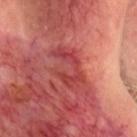follow-up: catalogued during a skin exam; not biopsied
image: total-body-photography crop, ~15 mm field of view
automated lesion analysis: a symmetry-axis asymmetry near 0.5; about 8 CIELAB-L* units darker than the surrounding skin; an automated nevus-likeness rating near 0 out of 100
illumination: cross-polarized illumination
anatomic site: the head or neck
subject: male, aged 58 to 62
lesion size: ~5 mm (longest diameter)